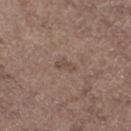follow-up: imaged on a skin check; not biopsied
tile lighting: white-light
lesion size: about 2.5 mm
automated lesion analysis: lesion-presence confidence of about 100/100
location: the left lower leg
subject: male, aged around 70
image: total-body-photography crop, ~15 mm field of view